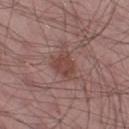follow-up=imaged on a skin check; not biopsied
lighting=white-light
anatomic site=the left thigh
image-analysis metrics=a footprint of about 7.5 mm², an eccentricity of roughly 0.75, and two-axis asymmetry of about 0.4; an average lesion color of about L≈44 a*≈21 b*≈22 (CIELAB) and a normalized border contrast of about 7; a border-irregularity index near 4.5/10, a within-lesion color-variation index near 2/10, and peripheral color asymmetry of about 0.5; an automated nevus-likeness rating near 50 out of 100 and a lesion-detection confidence of about 100/100
subject=male, approximately 55 years of age
image source=~15 mm tile from a whole-body skin photo